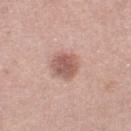workup = imaged on a skin check; not biopsied | anatomic site = the right thigh | patient = female, approximately 50 years of age | image = ~15 mm crop, total-body skin-cancer survey | image-analysis metrics = a footprint of about 8 mm² and an eccentricity of roughly 0.6; about 13 CIELAB-L* units darker than the surrounding skin and a normalized lesion–skin contrast near 8 | lighting = white-light illumination | diameter = ≈3.5 mm.